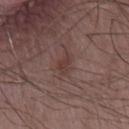Impression: The lesion was photographed on a routine skin check and not biopsied; there is no pathology result. Context: A male patient aged approximately 60. Automated tile analysis of the lesion measured a footprint of about 3 mm² and a shape-asymmetry score of about 0.35 (0 = symmetric). And it measured an automated nevus-likeness rating near 0 out of 100 and lesion-presence confidence of about 100/100. A roughly 15 mm field-of-view crop from a total-body skin photograph. Measured at roughly 3 mm in maximum diameter. On the chest.